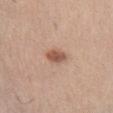biopsy status — no biopsy performed (imaged during a skin exam); lighting — white-light; image — 15 mm crop, total-body photography; subject — female, aged 23 to 27; anatomic site — the abdomen; size — about 3 mm.